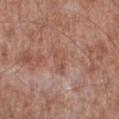Notes:
• workup — catalogued during a skin exam; not biopsied
• subject — male, aged approximately 55
• acquisition — 15 mm crop, total-body photography
• size — ≈3.5 mm
• site — the right lower leg